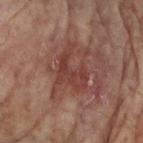{
  "biopsy_status": "not biopsied; imaged during a skin examination",
  "automated_metrics": {
    "area_mm2_approx": 10.0,
    "eccentricity": 0.75,
    "cielab_L": 35,
    "cielab_a": 22,
    "cielab_b": 22,
    "vs_skin_darker_L": 7.0,
    "vs_skin_contrast_norm": 6.5,
    "border_irregularity_0_10": 7.5,
    "color_variation_0_10": 3.0,
    "peripheral_color_asymmetry": 1.0
  },
  "lighting": "cross-polarized",
  "image": {
    "source": "total-body photography crop",
    "field_of_view_mm": 15
  },
  "patient": {
    "sex": "female",
    "age_approx": 80
  },
  "site": "left arm",
  "lesion_size": {
    "long_diameter_mm_approx": 5.0
  }
}